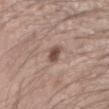No biopsy was performed on this lesion — it was imaged during a full skin examination and was not determined to be concerning. About 2.5 mm across. A male patient in their mid- to late 50s. Located on the arm. This image is a 15 mm lesion crop taken from a total-body photograph.